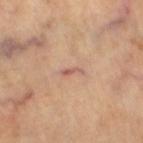Imaged during a routine full-body skin examination; the lesion was not biopsied and no histopathology is available.
Automated tile analysis of the lesion measured a shape eccentricity near 0.95.
This is a cross-polarized tile.
A roughly 15 mm field-of-view crop from a total-body skin photograph.
The lesion is located on the right thigh.
A female subject aged around 65.